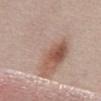Q: Is there a histopathology result?
A: no biopsy performed (imaged during a skin exam)
Q: How was the tile lit?
A: white-light
Q: What is the anatomic site?
A: the mid back
Q: How large is the lesion?
A: ≈9.5 mm
Q: How was this image acquired?
A: ~15 mm tile from a whole-body skin photo
Q: Automated lesion metrics?
A: a lesion area of about 36 mm², an outline eccentricity of about 0.8 (0 = round, 1 = elongated), and two-axis asymmetry of about 0.6; an average lesion color of about L≈60 a*≈16 b*≈25 (CIELAB), a lesion–skin lightness drop of about 8, and a normalized lesion–skin contrast near 5; a classifier nevus-likeness of about 65/100
Q: Who is the patient?
A: male, aged approximately 55